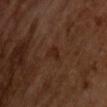Cropped from a whole-body photographic skin survey; the tile spans about 15 mm.
A male patient, approximately 65 years of age.
This is a cross-polarized tile.
An algorithmic analysis of the crop reported border irregularity of about 5 on a 0–10 scale, a color-variation rating of about 0/10, and peripheral color asymmetry of about 0. It also reported an automated nevus-likeness rating near 5 out of 100 and lesion-presence confidence of about 100/100.